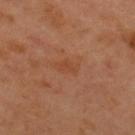| field | value |
|---|---|
| notes | imaged on a skin check; not biopsied |
| image source | total-body-photography crop, ~15 mm field of view |
| patient | male, aged 48 to 52 |
| site | the upper back |